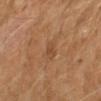Recorded during total-body skin imaging; not selected for excision or biopsy. The lesion-visualizer software estimated a lesion area of about 3 mm² and a shape-asymmetry score of about 0.3 (0 = symmetric). It also reported an average lesion color of about L≈43 a*≈19 b*≈31 (CIELAB), a lesion–skin lightness drop of about 6, and a lesion-to-skin contrast of about 5 (normalized; higher = more distinct). It also reported a border-irregularity rating of about 3/10, internal color variation of about 2 on a 0–10 scale, and a peripheral color-asymmetry measure near 0.5. It also reported an automated nevus-likeness rating near 0 out of 100 and lesion-presence confidence of about 100/100. The subject is a male roughly 60 years of age. A roughly 15 mm field-of-view crop from a total-body skin photograph. The lesion is on the left forearm. The recorded lesion diameter is about 3 mm. The tile uses cross-polarized illumination.